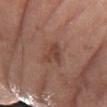Case summary:
* notes: catalogued during a skin exam; not biopsied
* body site: the arm
* patient: female, aged around 70
* image source: ~15 mm tile from a whole-body skin photo
* automated metrics: border irregularity of about 4.5 on a 0–10 scale and peripheral color asymmetry of about 0.5; a nevus-likeness score of about 0/100 and a lesion-detection confidence of about 100/100
* lighting: white-light illumination